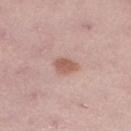follow-up — no biopsy performed (imaged during a skin exam)
illumination — white-light illumination
diameter — ~2.5 mm (longest diameter)
subject — female, aged 48 to 52
site — the left lower leg
automated metrics — an area of roughly 4.5 mm², an outline eccentricity of about 0.6 (0 = round, 1 = elongated), and a symmetry-axis asymmetry near 0.2; roughly 10 lightness units darker than nearby skin; a border-irregularity index near 2/10 and internal color variation of about 2 on a 0–10 scale; a classifier nevus-likeness of about 85/100 and a detector confidence of about 100 out of 100 that the crop contains a lesion
acquisition — 15 mm crop, total-body photography